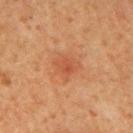  biopsy_status: not biopsied; imaged during a skin examination
  site: mid back
  image:
    source: total-body photography crop
    field_of_view_mm: 15
  lesion_size:
    long_diameter_mm_approx: 3.0
  automated_metrics:
    area_mm2_approx: 7.0
    shape_asymmetry: 0.25
    cielab_L: 46
    cielab_a: 25
    cielab_b: 33
    vs_skin_darker_L: 6.0
    vs_skin_contrast_norm: 5.0
    border_irregularity_0_10: 2.5
    color_variation_0_10: 4.0
    peripheral_color_asymmetry: 1.5
    nevus_likeness_0_100: 0
    lesion_detection_confidence_0_100: 100
  patient:
    sex: male
    age_approx: 65